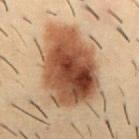- workup — total-body-photography surveillance lesion; no biopsy
- subject — male, about 30 years old
- diameter — ≈9 mm
- image — 15 mm crop, total-body photography
- illumination — cross-polarized
- site — the abdomen
- image-analysis metrics — a mean CIELAB color near L≈40 a*≈18 b*≈28; an automated nevus-likeness rating near 100 out of 100